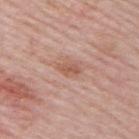Recorded during total-body skin imaging; not selected for excision or biopsy. Captured under white-light illumination. The total-body-photography lesion software estimated a lesion area of about 3 mm², an eccentricity of roughly 0.85, and a symmetry-axis asymmetry near 0.25. And it measured a mean CIELAB color near L≈56 a*≈22 b*≈29 and a normalized border contrast of about 6.5. A male subject roughly 65 years of age. Approximately 2.5 mm at its widest. A 15 mm close-up extracted from a 3D total-body photography capture. The lesion is on the upper back.